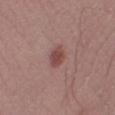<case>
<biopsy_status>not biopsied; imaged during a skin examination</biopsy_status>
<site>left lower leg</site>
<image>
  <source>total-body photography crop</source>
  <field_of_view_mm>15</field_of_view_mm>
</image>
<patient>
  <sex>male</sex>
  <age_approx>20</age_approx>
</patient>
</case>